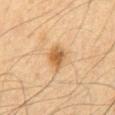The lesion was photographed on a routine skin check and not biopsied; there is no pathology result. Imaged with cross-polarized lighting. From the mid back. A male patient, aged 63–67. About 3 mm across. Cropped from a whole-body photographic skin survey; the tile spans about 15 mm. Automated tile analysis of the lesion measured an average lesion color of about L≈48 a*≈17 b*≈35 (CIELAB), a lesion–skin lightness drop of about 10, and a normalized border contrast of about 8.5.